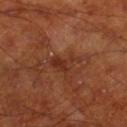The lesion was photographed on a routine skin check and not biopsied; there is no pathology result. Measured at roughly 3.5 mm in maximum diameter. The lesion is on the right lower leg. A 15 mm close-up tile from a total-body photography series done for melanoma screening. Captured under cross-polarized illumination. A male subject, aged around 70.